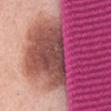This lesion was catalogued during total-body skin photography and was not selected for biopsy. Imaged with white-light lighting. Automated tile analysis of the lesion measured a lesion area of about 12 mm², an eccentricity of roughly 0.75, and two-axis asymmetry of about 0.55. And it measured border irregularity of about 7 on a 0–10 scale, a within-lesion color-variation index near 3.5/10, and a peripheral color-asymmetry measure near 1. A female patient, aged approximately 65. A lesion tile, about 15 mm wide, cut from a 3D total-body photograph. The lesion is located on the abdomen. Longest diameter approximately 5.5 mm.